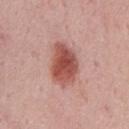Case summary:
– workup · imaged on a skin check; not biopsied
– image · total-body-photography crop, ~15 mm field of view
– patient · male, aged around 75
– body site · the front of the torso